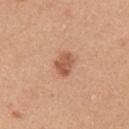follow-up = imaged on a skin check; not biopsied.